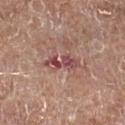Impression:
Part of a total-body skin-imaging series; this lesion was reviewed on a skin check and was not flagged for biopsy.
Context:
Imaged with white-light lighting. A male subject, aged 78 to 82. Cropped from a total-body skin-imaging series; the visible field is about 15 mm. Located on the right lower leg.